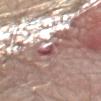Clinical impression:
Imaged during a routine full-body skin examination; the lesion was not biopsied and no histopathology is available.
Background:
The patient is a male roughly 80 years of age. On the chest. The tile uses white-light illumination. The lesion-visualizer software estimated a mean CIELAB color near L≈53 a*≈22 b*≈20, roughly 11 lightness units darker than nearby skin, and a normalized lesion–skin contrast near 7.5. A 15 mm close-up extracted from a 3D total-body photography capture.